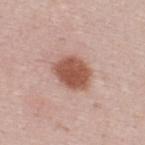biopsy_status: not biopsied; imaged during a skin examination
site: upper back
lesion_size:
  long_diameter_mm_approx: 4.5
lighting: white-light
automated_metrics:
  eccentricity: 0.65
  border_irregularity_0_10: 2.0
  color_variation_0_10: 2.5
  peripheral_color_asymmetry: 1.0
  nevus_likeness_0_100: 100
image:
  source: total-body photography crop
  field_of_view_mm: 15
patient:
  sex: male
  age_approx: 25